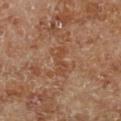  automated_metrics:
    area_mm2_approx: 5.0
    eccentricity: 0.95
    shape_asymmetry: 0.4
  patient:
    sex: male
    age_approx: 70
  image:
    source: total-body photography crop
    field_of_view_mm: 15
  lighting: cross-polarized
  lesion_size:
    long_diameter_mm_approx: 4.0
  site: leg